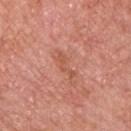Imaged during a routine full-body skin examination; the lesion was not biopsied and no histopathology is available.
This is a white-light tile.
A male patient aged approximately 70.
Automated image analysis of the tile measured a border-irregularity rating of about 8/10 and peripheral color asymmetry of about 0. The software also gave a classifier nevus-likeness of about 0/100 and a detector confidence of about 100 out of 100 that the crop contains a lesion.
The lesion is on the chest.
A close-up tile cropped from a whole-body skin photograph, about 15 mm across.
Approximately 3.5 mm at its widest.